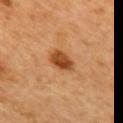Recorded during total-body skin imaging; not selected for excision or biopsy. The tile uses cross-polarized illumination. A 15 mm close-up extracted from a 3D total-body photography capture. Located on the upper back. Measured at roughly 3.5 mm in maximum diameter. A female subject approximately 65 years of age.